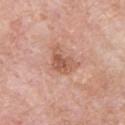{
  "biopsy_status": "not biopsied; imaged during a skin examination",
  "image": {
    "source": "total-body photography crop",
    "field_of_view_mm": 15
  },
  "lesion_size": {
    "long_diameter_mm_approx": 3.5
  },
  "patient": {
    "sex": "male",
    "age_approx": 55
  },
  "site": "chest",
  "automated_metrics": {
    "vs_skin_contrast_norm": 7.0,
    "color_variation_0_10": 4.0,
    "peripheral_color_asymmetry": 1.0
  }
}